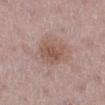  biopsy_status: not biopsied; imaged during a skin examination
  image:
    source: total-body photography crop
    field_of_view_mm: 15
  automated_metrics:
    cielab_L: 54
    cielab_a: 19
    cielab_b: 24
    vs_skin_darker_L: 8.0
    vs_skin_contrast_norm: 6.5
    color_variation_0_10: 3.0
    peripheral_color_asymmetry: 1.0
    nevus_likeness_0_100: 55
  patient:
    sex: female
    age_approx: 45
  lighting: white-light
  site: leg